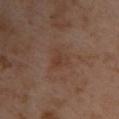No biopsy was performed on this lesion — it was imaged during a full skin examination and was not determined to be concerning. The lesion-visualizer software estimated a lesion area of about 5 mm², a shape eccentricity near 0.4, and a shape-asymmetry score of about 0.4 (0 = symmetric). The software also gave a lesion color around L≈39 a*≈18 b*≈27 in CIELAB and a lesion–skin lightness drop of about 5. It also reported border irregularity of about 3.5 on a 0–10 scale, a color-variation rating of about 2.5/10, and a peripheral color-asymmetry measure near 1. And it measured a nevus-likeness score of about 0/100. A 15 mm crop from a total-body photograph taken for skin-cancer surveillance. Longest diameter approximately 3 mm. From the upper back. A female subject, aged 38–42. Captured under cross-polarized illumination.